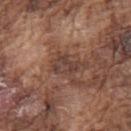* image: total-body-photography crop, ~15 mm field of view
* illumination: white-light illumination
* body site: the left upper arm
* TBP lesion metrics: a lesion area of about 5.5 mm², an eccentricity of roughly 0.7, and a symmetry-axis asymmetry near 0.35; an average lesion color of about L≈40 a*≈19 b*≈23 (CIELAB) and a lesion-to-skin contrast of about 7 (normalized; higher = more distinct); border irregularity of about 3.5 on a 0–10 scale and a peripheral color-asymmetry measure near 1; a nevus-likeness score of about 0/100
* lesion diameter: about 3 mm
* patient: male, roughly 75 years of age